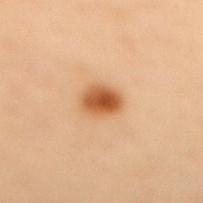Recorded during total-body skin imaging; not selected for excision or biopsy. A close-up tile cropped from a whole-body skin photograph, about 15 mm across. Approximately 3 mm at its widest. The lesion is located on the mid back. A female patient aged 48–52. Captured under cross-polarized illumination.